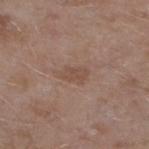Recorded during total-body skin imaging; not selected for excision or biopsy. Captured under white-light illumination. Automated image analysis of the tile measured a lesion area of about 4.5 mm², an eccentricity of roughly 0.8, and a symmetry-axis asymmetry near 0.3. The analysis additionally found a mean CIELAB color near L≈49 a*≈17 b*≈26, a lesion–skin lightness drop of about 7, and a lesion-to-skin contrast of about 5.5 (normalized; higher = more distinct). The analysis additionally found border irregularity of about 3.5 on a 0–10 scale, a color-variation rating of about 1/10, and radial color variation of about 0.5. The software also gave a classifier nevus-likeness of about 0/100 and lesion-presence confidence of about 100/100. A roughly 15 mm field-of-view crop from a total-body skin photograph. From the left lower leg. A male patient approximately 45 years of age.